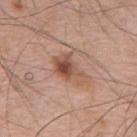Clinical impression:
Recorded during total-body skin imaging; not selected for excision or biopsy.
Acquisition and patient details:
A male subject, in their mid- to late 70s. A 15 mm close-up tile from a total-body photography series done for melanoma screening. The lesion-visualizer software estimated an area of roughly 7.5 mm², a shape eccentricity near 0.85, and two-axis asymmetry of about 0.4. The analysis additionally found a lesion color around L≈52 a*≈21 b*≈28 in CIELAB, roughly 11 lightness units darker than nearby skin, and a lesion-to-skin contrast of about 8 (normalized; higher = more distinct). The software also gave border irregularity of about 4.5 on a 0–10 scale, internal color variation of about 7 on a 0–10 scale, and radial color variation of about 2.5. And it measured a nevus-likeness score of about 50/100 and a detector confidence of about 100 out of 100 that the crop contains a lesion. The lesion is on the back. Imaged with white-light lighting. The lesion's longest dimension is about 4 mm.